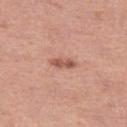Imaged during a routine full-body skin examination; the lesion was not biopsied and no histopathology is available. Captured under white-light illumination. A female subject aged 38–42. The total-body-photography lesion software estimated a border-irregularity rating of about 2.5/10 and peripheral color asymmetry of about 0.5. And it measured a nevus-likeness score of about 45/100 and lesion-presence confidence of about 100/100. Longest diameter approximately 3 mm. The lesion is located on the left thigh. A 15 mm crop from a total-body photograph taken for skin-cancer surveillance.